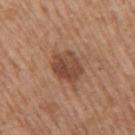Findings:
* workup: imaged on a skin check; not biopsied
* subject: male, in their mid- to late 60s
* acquisition: total-body-photography crop, ~15 mm field of view
* automated lesion analysis: a mean CIELAB color near L≈46 a*≈21 b*≈30, roughly 11 lightness units darker than nearby skin, and a normalized lesion–skin contrast near 8; radial color variation of about 1.5; a classifier nevus-likeness of about 70/100 and a detector confidence of about 100 out of 100 that the crop contains a lesion
* illumination: white-light
* lesion size: ~5 mm (longest diameter)
* location: the arm